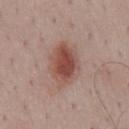Notes:
• biopsy status: total-body-photography surveillance lesion; no biopsy
• anatomic site: the mid back
• subject: male, aged 48–52
• TBP lesion metrics: a border-irregularity rating of about 2/10, a within-lesion color-variation index near 5.5/10, and radial color variation of about 1.5; a nevus-likeness score of about 100/100
• image: ~15 mm tile from a whole-body skin photo